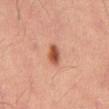Assessment:
The lesion was tiled from a total-body skin photograph and was not biopsied.
Context:
The lesion is on the abdomen. This is a cross-polarized tile. The patient is a male roughly 55 years of age. A close-up tile cropped from a whole-body skin photograph, about 15 mm across. Approximately 3 mm at its widest.